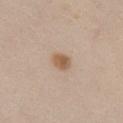Impression:
Imaged during a routine full-body skin examination; the lesion was not biopsied and no histopathology is available.
Clinical summary:
Captured under white-light illumination. From the chest. A region of skin cropped from a whole-body photographic capture, roughly 15 mm wide. The subject is a female approximately 40 years of age. Longest diameter approximately 2 mm.